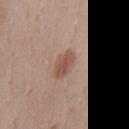The subject is a female roughly 40 years of age. Captured under white-light illumination. About 3.5 mm across. Located on the chest. Automated tile analysis of the lesion measured an average lesion color of about L≈52 a*≈19 b*≈28 (CIELAB) and a lesion–skin lightness drop of about 10. A lesion tile, about 15 mm wide, cut from a 3D total-body photograph.